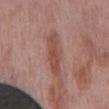Clinical impression:
No biopsy was performed on this lesion — it was imaged during a full skin examination and was not determined to be concerning.
Context:
A male subject aged 73–77. From the mid back. A 15 mm close-up tile from a total-body photography series done for melanoma screening.